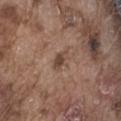Assessment: Part of a total-body skin-imaging series; this lesion was reviewed on a skin check and was not flagged for biopsy. Context: Automated tile analysis of the lesion measured a mean CIELAB color near L≈44 a*≈17 b*≈26 and a normalized lesion–skin contrast near 7.5. It also reported a border-irregularity rating of about 3/10, internal color variation of about 1.5 on a 0–10 scale, and radial color variation of about 0.5. On the abdomen. The lesion's longest dimension is about 2.5 mm. A male subject, aged around 75. This image is a 15 mm lesion crop taken from a total-body photograph.